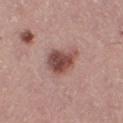Part of a total-body skin-imaging series; this lesion was reviewed on a skin check and was not flagged for biopsy. Imaged with white-light lighting. From the right thigh. Cropped from a whole-body photographic skin survey; the tile spans about 15 mm. A female patient, roughly 40 years of age. Longest diameter approximately 4.5 mm.